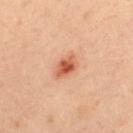The lesion was photographed on a routine skin check and not biopsied; there is no pathology result. The lesion is located on the back. The subject is a male about 35 years old. Cropped from a total-body skin-imaging series; the visible field is about 15 mm.